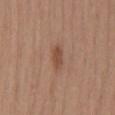{"biopsy_status": "not biopsied; imaged during a skin examination", "site": "mid back", "patient": {"sex": "female", "age_approx": 55}, "image": {"source": "total-body photography crop", "field_of_view_mm": 15}}